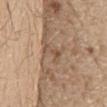notes: catalogued during a skin exam; not biopsied
subject: male, aged around 70
location: the chest
image source: total-body-photography crop, ~15 mm field of view